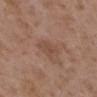biopsy_status: not biopsied; imaged during a skin examination
site: upper back
image:
  source: total-body photography crop
  field_of_view_mm: 15
lesion_size:
  long_diameter_mm_approx: 2.5
lighting: white-light
patient:
  sex: female
  age_approx: 35